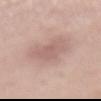Recorded during total-body skin imaging; not selected for excision or biopsy.
Cropped from a total-body skin-imaging series; the visible field is about 15 mm.
From the abdomen.
A female subject in their 40s.
Captured under white-light illumination.